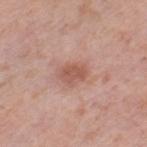biopsy status = no biopsy performed (imaged during a skin exam) | image-analysis metrics = an area of roughly 5.5 mm² and a shape eccentricity near 0.7; a peripheral color-asymmetry measure near 0.5; an automated nevus-likeness rating near 45 out of 100 and a detector confidence of about 100 out of 100 that the crop contains a lesion | diameter = about 3 mm | subject = female, in their 40s | site = the left thigh | acquisition = total-body-photography crop, ~15 mm field of view.